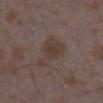<record>
  <biopsy_status>not biopsied; imaged during a skin examination</biopsy_status>
  <image>
    <source>total-body photography crop</source>
    <field_of_view_mm>15</field_of_view_mm>
  </image>
  <site>right lower leg</site>
  <patient>
    <sex>female</sex>
    <age_approx>35</age_approx>
  </patient>
</record>